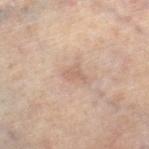Case summary:
• biopsy status: no biopsy performed (imaged during a skin exam)
• location: the leg
• automated lesion analysis: an area of roughly 3 mm², an outline eccentricity of about 0.75 (0 = round, 1 = elongated), and two-axis asymmetry of about 0.45; border irregularity of about 4 on a 0–10 scale and a within-lesion color-variation index near 1/10; an automated nevus-likeness rating near 0 out of 100 and a lesion-detection confidence of about 100/100
• diameter: ~2.5 mm (longest diameter)
• patient: female, aged around 50
• tile lighting: cross-polarized illumination
• acquisition: ~15 mm tile from a whole-body skin photo